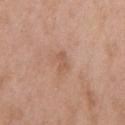Recorded during total-body skin imaging; not selected for excision or biopsy. A male patient in their 50s. This is a white-light tile. The lesion-visualizer software estimated an area of roughly 3.5 mm², an eccentricity of roughly 0.75, and two-axis asymmetry of about 0.3. The software also gave an average lesion color of about L≈57 a*≈20 b*≈31 (CIELAB), roughly 7 lightness units darker than nearby skin, and a normalized lesion–skin contrast near 5. And it measured a border-irregularity index near 3/10, a within-lesion color-variation index near 2/10, and peripheral color asymmetry of about 1. On the front of the torso. A 15 mm close-up extracted from a 3D total-body photography capture.